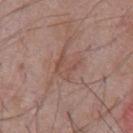{"biopsy_status": "not biopsied; imaged during a skin examination", "site": "chest", "lighting": "white-light", "image": {"source": "total-body photography crop", "field_of_view_mm": 15}, "lesion_size": {"long_diameter_mm_approx": 3.0}, "patient": {"sex": "male", "age_approx": 60}}